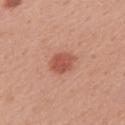| field | value |
|---|---|
| notes | total-body-photography surveillance lesion; no biopsy |